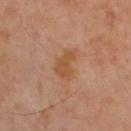Recorded during total-body skin imaging; not selected for excision or biopsy.
Located on the back.
This is a cross-polarized tile.
Cropped from a whole-body photographic skin survey; the tile spans about 15 mm.
A male subject approximately 50 years of age.
The lesion's longest dimension is about 3.5 mm.